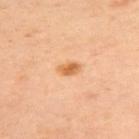Assessment: This lesion was catalogued during total-body skin photography and was not selected for biopsy. Context: Approximately 2.5 mm at its widest. The lesion is on the upper back. Imaged with cross-polarized lighting. A lesion tile, about 15 mm wide, cut from a 3D total-body photograph. A female subject aged approximately 40.